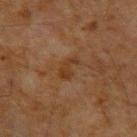No biopsy was performed on this lesion — it was imaged during a full skin examination and was not determined to be concerning. Imaged with cross-polarized lighting. A male subject, about 50 years old. Longest diameter approximately 3 mm. A 15 mm crop from a total-body photograph taken for skin-cancer surveillance. The lesion is located on the left upper arm. The total-body-photography lesion software estimated a lesion area of about 4.5 mm², an eccentricity of roughly 0.85, and a shape-asymmetry score of about 0.45 (0 = symmetric).